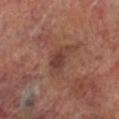The lesion was tiled from a total-body skin photograph and was not biopsied. Located on the right lower leg. A roughly 15 mm field-of-view crop from a total-body skin photograph. The tile uses cross-polarized illumination. Automated tile analysis of the lesion measured a shape-asymmetry score of about 0.35 (0 = symmetric). The analysis additionally found a mean CIELAB color near L≈38 a*≈21 b*≈24, a lesion–skin lightness drop of about 8, and a lesion-to-skin contrast of about 7 (normalized; higher = more distinct). The analysis additionally found a border-irregularity rating of about 3.5/10 and peripheral color asymmetry of about 0.5. The analysis additionally found a lesion-detection confidence of about 100/100. A male patient, aged 68–72. About 3 mm across.